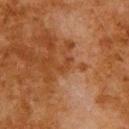{
  "biopsy_status": "not biopsied; imaged during a skin examination",
  "image": {
    "source": "total-body photography crop",
    "field_of_view_mm": 15
  },
  "lighting": "cross-polarized",
  "lesion_size": {
    "long_diameter_mm_approx": 2.5
  },
  "patient": {
    "sex": "male",
    "age_approx": 80
  },
  "site": "upper back",
  "automated_metrics": {
    "area_mm2_approx": 2.0,
    "vs_skin_contrast_norm": 6.5,
    "border_irregularity_0_10": 7.0,
    "color_variation_0_10": 0.0,
    "peripheral_color_asymmetry": 0.0,
    "nevus_likeness_0_100": 0,
    "lesion_detection_confidence_0_100": 100
  }
}